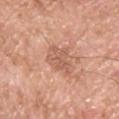biopsy_status: not biopsied; imaged during a skin examination
patient:
  sex: male
  age_approx: 55
lighting: white-light
lesion_size:
  long_diameter_mm_approx: 3.0
image:
  source: total-body photography crop
  field_of_view_mm: 15
automated_metrics:
  eccentricity: 0.25
  shape_asymmetry: 0.3
  border_irregularity_0_10: 4.5
  color_variation_0_10: 2.5
  peripheral_color_asymmetry: 1.0
  lesion_detection_confidence_0_100: 100
site: chest